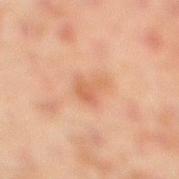Clinical impression: No biopsy was performed on this lesion — it was imaged during a full skin examination and was not determined to be concerning. Image and clinical context: Cropped from a total-body skin-imaging series; the visible field is about 15 mm. The total-body-photography lesion software estimated a lesion area of about 5 mm², a shape eccentricity near 0.75, and two-axis asymmetry of about 0.45. It also reported a lesion color around L≈54 a*≈22 b*≈32 in CIELAB. The patient is a male roughly 45 years of age. This is a cross-polarized tile. The lesion is on the right lower leg.